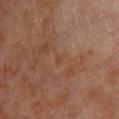Imaged during a routine full-body skin examination; the lesion was not biopsied and no histopathology is available. The total-body-photography lesion software estimated a symmetry-axis asymmetry near 0.3. And it measured an automated nevus-likeness rating near 0 out of 100 and a lesion-detection confidence of about 100/100. On the left lower leg. A close-up tile cropped from a whole-body skin photograph, about 15 mm across. The patient is a male aged 68–72. Longest diameter approximately 1.5 mm. This is a cross-polarized tile.